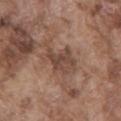Captured during whole-body skin photography for melanoma surveillance; the lesion was not biopsied. A roughly 15 mm field-of-view crop from a total-body skin photograph. Longest diameter approximately 5.5 mm. Automated tile analysis of the lesion measured a lesion area of about 11 mm², an outline eccentricity of about 0.8 (0 = round, 1 = elongated), and two-axis asymmetry of about 0.4. The analysis additionally found a nevus-likeness score of about 0/100 and a lesion-detection confidence of about 75/100. On the mid back. A male patient, about 75 years old.